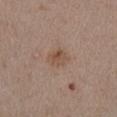workup=no biopsy performed (imaged during a skin exam)
lesion size=≈3 mm
site=the arm
image-analysis metrics=roughly 7 lightness units darker than nearby skin and a normalized lesion–skin contrast near 6.5; a border-irregularity rating of about 1.5/10 and peripheral color asymmetry of about 1.5; an automated nevus-likeness rating near 10 out of 100 and lesion-presence confidence of about 100/100
patient=male, aged 53–57
image=15 mm crop, total-body photography
lighting=white-light illumination